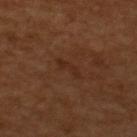Q: Was a biopsy performed?
A: imaged on a skin check; not biopsied
Q: What lighting was used for the tile?
A: cross-polarized
Q: What are the patient's age and sex?
A: male, in their mid-60s
Q: What is the imaging modality?
A: 15 mm crop, total-body photography